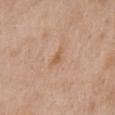| key | value |
|---|---|
| follow-up | catalogued during a skin exam; not biopsied |
| illumination | white-light |
| patient | male, approximately 65 years of age |
| lesion size | ≈2.5 mm |
| anatomic site | the chest |
| image source | total-body-photography crop, ~15 mm field of view |